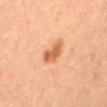<case>
<biopsy_status>not biopsied; imaged during a skin examination</biopsy_status>
<automated_metrics>
  <border_irregularity_0_10>3.0</border_irregularity_0_10>
  <color_variation_0_10>2.5</color_variation_0_10>
  <peripheral_color_asymmetry>1.0</peripheral_color_asymmetry>
  <lesion_detection_confidence_0_100>100</lesion_detection_confidence_0_100>
</automated_metrics>
<patient>
  <sex>female</sex>
  <age_approx>60</age_approx>
</patient>
<lighting>cross-polarized</lighting>
<site>mid back</site>
<lesion_size>
  <long_diameter_mm_approx>3.5</long_diameter_mm_approx>
</lesion_size>
<image>
  <source>total-body photography crop</source>
  <field_of_view_mm>15</field_of_view_mm>
</image>
</case>